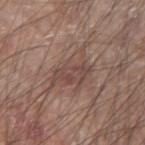Q: How was the tile lit?
A: white-light illumination
Q: How was this image acquired?
A: ~15 mm crop, total-body skin-cancer survey
Q: Lesion size?
A: ≈7 mm
Q: What is the anatomic site?
A: the left forearm
Q: Automated lesion metrics?
A: a footprint of about 14 mm², an eccentricity of roughly 0.75, and a shape-asymmetry score of about 0.5 (0 = symmetric); border irregularity of about 7 on a 0–10 scale, internal color variation of about 4 on a 0–10 scale, and a peripheral color-asymmetry measure near 1.5
Q: Patient demographics?
A: male, roughly 60 years of age